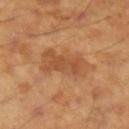| feature | finding |
|---|---|
| biopsy status | no biopsy performed (imaged during a skin exam) |
| lesion size | about 6 mm |
| patient | male, aged 43 to 47 |
| location | the left forearm |
| imaging modality | total-body-photography crop, ~15 mm field of view |
| illumination | cross-polarized |